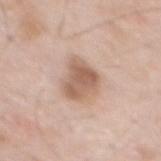Assessment: Imaged during a routine full-body skin examination; the lesion was not biopsied and no histopathology is available. Background: A male subject approximately 60 years of age. Imaged with white-light lighting. Measured at roughly 4.5 mm in maximum diameter. On the back. A lesion tile, about 15 mm wide, cut from a 3D total-body photograph.